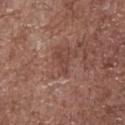notes=total-body-photography surveillance lesion; no biopsy
patient=male, approximately 70 years of age
image source=15 mm crop, total-body photography
site=the abdomen
automated lesion analysis=an average lesion color of about L≈43 a*≈23 b*≈25 (CIELAB), roughly 7 lightness units darker than nearby skin, and a normalized lesion–skin contrast near 5.5
illumination=white-light illumination
lesion size=~2.5 mm (longest diameter)